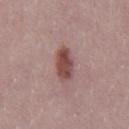biopsy status — imaged on a skin check; not biopsied | diameter — ~4.5 mm (longest diameter) | subject — male, roughly 30 years of age | imaging modality — ~15 mm crop, total-body skin-cancer survey.